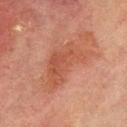The lesion was tiled from a total-body skin photograph and was not biopsied. The lesion's longest dimension is about 7 mm. Automated tile analysis of the lesion measured a footprint of about 17 mm², an eccentricity of roughly 0.85, and two-axis asymmetry of about 0.45. The software also gave an average lesion color of about L≈43 a*≈23 b*≈28 (CIELAB), roughly 7 lightness units darker than nearby skin, and a lesion-to-skin contrast of about 6 (normalized; higher = more distinct). The analysis additionally found a border-irregularity index near 5.5/10, a color-variation rating of about 3/10, and radial color variation of about 1. A male subject roughly 70 years of age. The lesion is on the chest. A region of skin cropped from a whole-body photographic capture, roughly 15 mm wide.